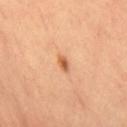A close-up tile cropped from a whole-body skin photograph, about 15 mm across. Approximately 2 mm at its widest. The lesion is on the right thigh. The patient is a female aged 53–57. The total-body-photography lesion software estimated an average lesion color of about L≈62 a*≈27 b*≈41 (CIELAB), roughly 12 lightness units darker than nearby skin, and a normalized lesion–skin contrast near 8. The analysis additionally found border irregularity of about 2 on a 0–10 scale, a within-lesion color-variation index near 2/10, and peripheral color asymmetry of about 0.5. Captured under cross-polarized illumination.